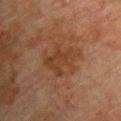Case summary:
– follow-up · catalogued during a skin exam; not biopsied
– imaging modality · ~15 mm crop, total-body skin-cancer survey
– patient · male, approximately 75 years of age
– TBP lesion metrics · a footprint of about 12 mm² and a shape-asymmetry score of about 0.4 (0 = symmetric); a mean CIELAB color near L≈33 a*≈19 b*≈28 and a normalized border contrast of about 6; a border-irregularity index near 5.5/10, a within-lesion color-variation index near 3/10, and a peripheral color-asymmetry measure near 1
– tile lighting · cross-polarized illumination
– site · the chest